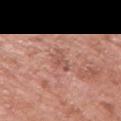The lesion was tiled from a total-body skin photograph and was not biopsied. A 15 mm close-up tile from a total-body photography series done for melanoma screening. A male subject about 70 years old. Automated tile analysis of the lesion measured a lesion area of about 4 mm² and a shape-asymmetry score of about 0.4 (0 = symmetric). The software also gave about 8 CIELAB-L* units darker than the surrounding skin and a lesion-to-skin contrast of about 5.5 (normalized; higher = more distinct). The analysis additionally found a border-irregularity index near 5/10, a color-variation rating of about 1.5/10, and radial color variation of about 0.5. It also reported an automated nevus-likeness rating near 0 out of 100 and a detector confidence of about 100 out of 100 that the crop contains a lesion. The lesion is on the right upper arm. Measured at roughly 3.5 mm in maximum diameter.